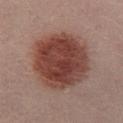About 7 mm across.
On the left lower leg.
This image is a 15 mm lesion crop taken from a total-body photograph.
A male patient aged 38–42.